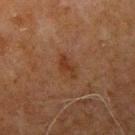workup=no biopsy performed (imaged during a skin exam); diameter=about 3.5 mm; image source=~15 mm tile from a whole-body skin photo; body site=the left upper arm; lighting=cross-polarized; subject=male, aged 78 to 82.